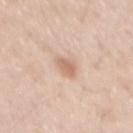{"biopsy_status": "not biopsied; imaged during a skin examination", "lighting": "white-light", "image": {"source": "total-body photography crop", "field_of_view_mm": 15}, "patient": {"sex": "male", "age_approx": 60}, "site": "mid back", "lesion_size": {"long_diameter_mm_approx": 2.5}, "automated_metrics": {"cielab_L": 65, "cielab_a": 19, "cielab_b": 30, "vs_skin_darker_L": 10.0, "vs_skin_contrast_norm": 6.5, "border_irregularity_0_10": 1.5, "color_variation_0_10": 1.5, "peripheral_color_asymmetry": 0.5, "lesion_detection_confidence_0_100": 100}}